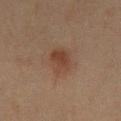Imaged during a routine full-body skin examination; the lesion was not biopsied and no histopathology is available. The recorded lesion diameter is about 3.5 mm. The subject is a male about 45 years old. The total-body-photography lesion software estimated a mean CIELAB color near L≈33 a*≈16 b*≈24, roughly 7 lightness units darker than nearby skin, and a normalized border contrast of about 7. And it measured a nevus-likeness score of about 65/100. This image is a 15 mm lesion crop taken from a total-body photograph. The lesion is located on the chest. The tile uses cross-polarized illumination.